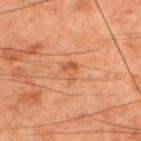  biopsy_status: not biopsied; imaged during a skin examination
  patient:
    sex: male
    age_approx: 60
  lesion_size:
    long_diameter_mm_approx: 2.5
  image:
    source: total-body photography crop
    field_of_view_mm: 15
  site: upper back